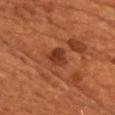Q: Was a biopsy performed?
A: total-body-photography surveillance lesion; no biopsy
Q: Where on the body is the lesion?
A: the chest
Q: How was the tile lit?
A: cross-polarized
Q: Patient demographics?
A: male, in their mid- to late 50s
Q: What did automated image analysis measure?
A: a footprint of about 5 mm² and two-axis asymmetry of about 0.3
Q: What is the imaging modality?
A: 15 mm crop, total-body photography
Q: Lesion size?
A: about 3 mm